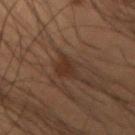Imaged during a routine full-body skin examination; the lesion was not biopsied and no histopathology is available.
The patient is a male in their mid-50s.
A 15 mm close-up tile from a total-body photography series done for melanoma screening.
From the arm.